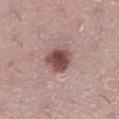Case summary:
* follow-up: no biopsy performed (imaged during a skin exam)
* image source: ~15 mm tile from a whole-body skin photo
* patient: female, aged approximately 40
* automated metrics: an area of roughly 9.5 mm², an eccentricity of roughly 0.55, and a symmetry-axis asymmetry near 0.2; a classifier nevus-likeness of about 90/100
* body site: the right lower leg
* lighting: white-light illumination